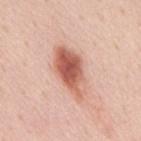  biopsy_status: not biopsied; imaged during a skin examination
  image:
    source: total-body photography crop
    field_of_view_mm: 15
  lesion_size:
    long_diameter_mm_approx: 6.0
  site: mid back
  lighting: white-light
  patient:
    sex: male
    age_approx: 50